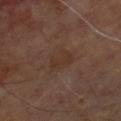The lesion was photographed on a routine skin check and not biopsied; there is no pathology result.
This is a cross-polarized tile.
A male patient in their 70s.
The recorded lesion diameter is about 3.5 mm.
The total-body-photography lesion software estimated a mean CIELAB color near L≈32 a*≈16 b*≈24 and roughly 4 lightness units darker than nearby skin. The software also gave a nevus-likeness score of about 0/100 and a lesion-detection confidence of about 100/100.
From the chest.
A 15 mm crop from a total-body photograph taken for skin-cancer surveillance.